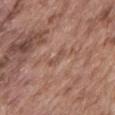Impression: Imaged during a routine full-body skin examination; the lesion was not biopsied and no histopathology is available. Image and clinical context: The lesion is on the abdomen. A male subject aged around 75. Longest diameter approximately 2.5 mm. A region of skin cropped from a whole-body photographic capture, roughly 15 mm wide.